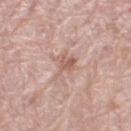<tbp_lesion>
  <biopsy_status>not biopsied; imaged during a skin examination</biopsy_status>
  <patient>
    <sex>female</sex>
    <age_approx>65</age_approx>
  </patient>
  <lesion_size>
    <long_diameter_mm_approx>4.0</long_diameter_mm_approx>
  </lesion_size>
  <site>left thigh</site>
  <automated_metrics>
    <area_mm2_approx>6.5</area_mm2_approx>
    <eccentricity>0.8</eccentricity>
    <shape_asymmetry>0.5</shape_asymmetry>
    <vs_skin_darker_L>8.0</vs_skin_darker_L>
    <vs_skin_contrast_norm>5.5</vs_skin_contrast_norm>
  </automated_metrics>
  <image>
    <source>total-body photography crop</source>
    <field_of_view_mm>15</field_of_view_mm>
  </image>
  <lighting>white-light</lighting>
</tbp_lesion>